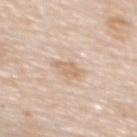Imaged during a routine full-body skin examination; the lesion was not biopsied and no histopathology is available. A close-up tile cropped from a whole-body skin photograph, about 15 mm across. Imaged with white-light lighting. A female patient roughly 50 years of age. On the upper back. Measured at roughly 2.5 mm in maximum diameter.